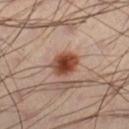This lesion was catalogued during total-body skin photography and was not selected for biopsy. Imaged with cross-polarized lighting. Automated image analysis of the tile measured a lesion area of about 7.5 mm², an outline eccentricity of about 0.55 (0 = round, 1 = elongated), and a shape-asymmetry score of about 0.15 (0 = symmetric). And it measured a lesion–skin lightness drop of about 15 and a normalized border contrast of about 12. The software also gave a border-irregularity index near 1.5/10, a color-variation rating of about 5.5/10, and peripheral color asymmetry of about 1.5. The software also gave an automated nevus-likeness rating near 100 out of 100 and a lesion-detection confidence of about 100/100. From the leg. Longest diameter approximately 3.5 mm. A roughly 15 mm field-of-view crop from a total-body skin photograph. A male patient, roughly 40 years of age.